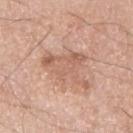biopsy status: catalogued during a skin exam; not biopsied | tile lighting: white-light illumination | image-analysis metrics: an outline eccentricity of about 0.7 (0 = round, 1 = elongated) and two-axis asymmetry of about 0.6; an automated nevus-likeness rating near 0 out of 100 and a detector confidence of about 100 out of 100 that the crop contains a lesion | image: 15 mm crop, total-body photography | diameter: ~6 mm (longest diameter) | anatomic site: the left upper arm | subject: male, in their mid-60s.